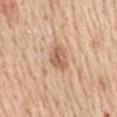Captured during whole-body skin photography for melanoma surveillance; the lesion was not biopsied. A region of skin cropped from a whole-body photographic capture, roughly 15 mm wide. About 3.5 mm across. Captured under white-light illumination. Located on the mid back. A male subject, roughly 60 years of age.